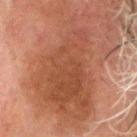diameter — ~14 mm (longest diameter) | patient — male, aged 78–82 | illumination — cross-polarized | image-analysis metrics — a footprint of about 65 mm², an outline eccentricity of about 0.85 (0 = round, 1 = elongated), and a symmetry-axis asymmetry near 0.4; a mean CIELAB color near L≈38 a*≈20 b*≈27 and about 8 CIELAB-L* units darker than the surrounding skin; a within-lesion color-variation index near 4/10 and a peripheral color-asymmetry measure near 1.5 | anatomic site — the head or neck | image source — ~15 mm crop, total-body skin-cancer survey.